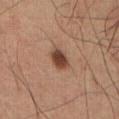No biopsy was performed on this lesion — it was imaged during a full skin examination and was not determined to be concerning. Cropped from a total-body skin-imaging series; the visible field is about 15 mm. The recorded lesion diameter is about 3 mm. The patient is a male aged approximately 60. Imaged with cross-polarized lighting.